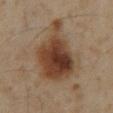Findings:
* imaging modality: 15 mm crop, total-body photography
* subject: male, in their 60s
* location: the abdomen